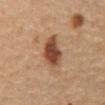Impression: No biopsy was performed on this lesion — it was imaged during a full skin examination and was not determined to be concerning. Background: The patient is a male approximately 65 years of age. An algorithmic analysis of the crop reported an area of roughly 9 mm², an eccentricity of roughly 0.85, and a symmetry-axis asymmetry near 0.25. It also reported a classifier nevus-likeness of about 90/100. A region of skin cropped from a whole-body photographic capture, roughly 15 mm wide. Measured at roughly 4.5 mm in maximum diameter. Captured under cross-polarized illumination. From the front of the torso.